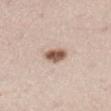Q: Is there a histopathology result?
A: total-body-photography surveillance lesion; no biopsy
Q: Lesion location?
A: the right thigh
Q: How was this image acquired?
A: total-body-photography crop, ~15 mm field of view
Q: How large is the lesion?
A: ≈3.5 mm
Q: What are the patient's age and sex?
A: male, aged 63 to 67
Q: How was the tile lit?
A: white-light illumination
Q: What did automated image analysis measure?
A: a lesion area of about 5.5 mm²; a nevus-likeness score of about 100/100 and a detector confidence of about 100 out of 100 that the crop contains a lesion